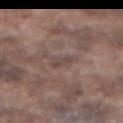Image and clinical context:
A close-up tile cropped from a whole-body skin photograph, about 15 mm across. The patient is a male roughly 75 years of age. On the abdomen. Captured under white-light illumination. Measured at roughly 2.5 mm in maximum diameter.